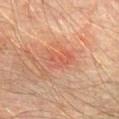Part of a total-body skin-imaging series; this lesion was reviewed on a skin check and was not flagged for biopsy. The patient is a male aged 73–77. Cropped from a total-body skin-imaging series; the visible field is about 15 mm. The lesion's longest dimension is about 4 mm. Automated tile analysis of the lesion measured a mean CIELAB color near L≈48 a*≈25 b*≈28, roughly 6 lightness units darker than nearby skin, and a normalized border contrast of about 4.5. And it measured a border-irregularity index near 3/10 and a peripheral color-asymmetry measure near 2. It also reported a classifier nevus-likeness of about 0/100 and a detector confidence of about 100 out of 100 that the crop contains a lesion. On the abdomen.